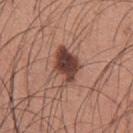<record>
<biopsy_status>not biopsied; imaged during a skin examination</biopsy_status>
<patient>
  <sex>male</sex>
  <age_approx>35</age_approx>
</patient>
<image>
  <source>total-body photography crop</source>
  <field_of_view_mm>15</field_of_view_mm>
</image>
<site>arm</site>
<automated_metrics>
  <cielab_L>43</cielab_L>
  <cielab_a>21</cielab_a>
  <cielab_b>25</cielab_b>
  <vs_skin_darker_L>16.0</vs_skin_darker_L>
  <color_variation_0_10>6.5</color_variation_0_10>
  <peripheral_color_asymmetry>2.0</peripheral_color_asymmetry>
  <lesion_detection_confidence_0_100>100</lesion_detection_confidence_0_100>
</automated_metrics>
</record>